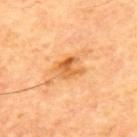Recorded during total-body skin imaging; not selected for excision or biopsy.
Approximately 3 mm at its widest.
The subject is a male aged 63–67.
The lesion is on the upper back.
A 15 mm close-up tile from a total-body photography series done for melanoma screening.
This is a cross-polarized tile.